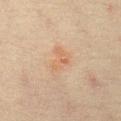location = the front of the torso; lighting = cross-polarized illumination; lesion diameter = ~3.5 mm (longest diameter); image = ~15 mm tile from a whole-body skin photo; subject = female, aged approximately 65.